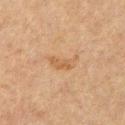Clinical impression:
Recorded during total-body skin imaging; not selected for excision or biopsy.
Context:
A lesion tile, about 15 mm wide, cut from a 3D total-body photograph. A male patient roughly 65 years of age. From the upper back.